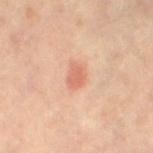{
  "biopsy_status": "not biopsied; imaged during a skin examination",
  "automated_metrics": {
    "area_mm2_approx": 4.5,
    "shape_asymmetry": 0.25,
    "cielab_L": 63,
    "cielab_a": 26,
    "cielab_b": 32,
    "vs_skin_darker_L": 9.0,
    "vs_skin_contrast_norm": 6.0,
    "border_irregularity_0_10": 2.0,
    "peripheral_color_asymmetry": 0.5
  },
  "site": "right leg",
  "lighting": "cross-polarized",
  "patient": {
    "sex": "female",
    "age_approx": 65
  },
  "image": {
    "source": "total-body photography crop",
    "field_of_view_mm": 15
  },
  "lesion_size": {
    "long_diameter_mm_approx": 2.5
  }
}